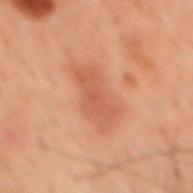Captured during whole-body skin photography for melanoma surveillance; the lesion was not biopsied. The tile uses cross-polarized illumination. A roughly 15 mm field-of-view crop from a total-body skin photograph. About 6.5 mm across. The lesion is on the mid back. The subject is a male aged around 60.